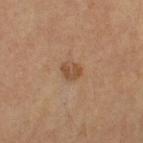| key | value |
|---|---|
| biopsy status | catalogued during a skin exam; not biopsied |
| TBP lesion metrics | a mean CIELAB color near L≈47 a*≈18 b*≈31, roughly 8 lightness units darker than nearby skin, and a normalized border contrast of about 6.5; a nevus-likeness score of about 50/100 and lesion-presence confidence of about 100/100 |
| acquisition | ~15 mm crop, total-body skin-cancer survey |
| diameter | about 2.5 mm |
| patient | female, aged around 65 |
| location | the leg |
| illumination | cross-polarized |